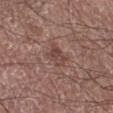Part of a total-body skin-imaging series; this lesion was reviewed on a skin check and was not flagged for biopsy.
Measured at roughly 3 mm in maximum diameter.
Imaged with white-light lighting.
The total-body-photography lesion software estimated an outline eccentricity of about 0.6 (0 = round, 1 = elongated) and a symmetry-axis asymmetry near 0.25. The analysis additionally found a mean CIELAB color near L≈44 a*≈19 b*≈22, a lesion–skin lightness drop of about 8, and a normalized border contrast of about 6.
The patient is a male approximately 75 years of age.
A roughly 15 mm field-of-view crop from a total-body skin photograph.
Located on the right lower leg.